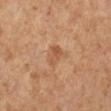<record>
  <biopsy_status>not biopsied; imaged during a skin examination</biopsy_status>
  <site>leg</site>
  <lesion_size>
    <long_diameter_mm_approx>3.0</long_diameter_mm_approx>
  </lesion_size>
  <patient>
    <sex>female</sex>
    <age_approx>65</age_approx>
  </patient>
  <lighting>cross-polarized</lighting>
  <image>
    <source>total-body photography crop</source>
    <field_of_view_mm>15</field_of_view_mm>
  </image>
  <automated_metrics>
    <area_mm2_approx>5.0</area_mm2_approx>
    <eccentricity>0.75</eccentricity>
    <shape_asymmetry>0.3</shape_asymmetry>
    <border_irregularity_0_10>3.5</border_irregularity_0_10>
    <color_variation_0_10>3.0</color_variation_0_10>
    <peripheral_color_asymmetry>1.0</peripheral_color_asymmetry>
    <nevus_likeness_0_100>0</nevus_likeness_0_100>
  </automated_metrics>
</record>